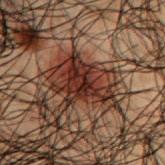  biopsy_status: not biopsied; imaged during a skin examination
  lesion_size:
    long_diameter_mm_approx: 5.5
  patient:
    sex: male
    age_approx: 50
  automated_metrics:
    cielab_L: 21
    cielab_a: 18
    cielab_b: 19
    vs_skin_darker_L: 11.0
    vs_skin_contrast_norm: 12.5
    nevus_likeness_0_100: 95
    lesion_detection_confidence_0_100: 80
  image:
    source: total-body photography crop
    field_of_view_mm: 15
  site: arm
  lighting: cross-polarized